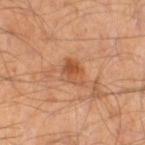workup: imaged on a skin check; not biopsied | patient: male, in their mid-40s | site: the right thigh | image source: ~15 mm crop, total-body skin-cancer survey | lesion diameter: ≈3 mm.